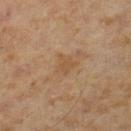Clinical impression:
Part of a total-body skin-imaging series; this lesion was reviewed on a skin check and was not flagged for biopsy.
Acquisition and patient details:
The recorded lesion diameter is about 2.5 mm. A male patient, approximately 60 years of age. This is a cross-polarized tile. Automated image analysis of the tile measured a lesion area of about 4.5 mm², an eccentricity of roughly 0.55, and two-axis asymmetry of about 0.35. The software also gave about 5 CIELAB-L* units darker than the surrounding skin and a lesion-to-skin contrast of about 5 (normalized; higher = more distinct). The analysis additionally found a border-irregularity index near 3.5/10 and a peripheral color-asymmetry measure near 0.5. On the left lower leg. A region of skin cropped from a whole-body photographic capture, roughly 15 mm wide.